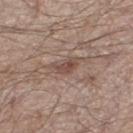workup=imaged on a skin check; not biopsied | acquisition=15 mm crop, total-body photography | lighting=white-light | site=the left thigh | lesion size=≈3 mm | automated metrics=an eccentricity of roughly 0.8 and two-axis asymmetry of about 0.4; border irregularity of about 4.5 on a 0–10 scale and radial color variation of about 1 | patient=male, about 55 years old.